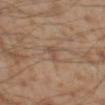Imaged during a routine full-body skin examination; the lesion was not biopsied and no histopathology is available.
The recorded lesion diameter is about 2.5 mm.
The lesion is located on the leg.
Automated image analysis of the tile measured a mean CIELAB color near L≈49 a*≈17 b*≈26, a lesion–skin lightness drop of about 6, and a normalized lesion–skin contrast near 5.
A close-up tile cropped from a whole-body skin photograph, about 15 mm across.
A male patient in their mid-50s.